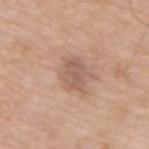Case summary:
– notes — catalogued during a skin exam; not biopsied
– illumination — white-light
– patient — male, roughly 65 years of age
– location — the upper back
– lesion size — ≈3.5 mm
– acquisition — ~15 mm tile from a whole-body skin photo